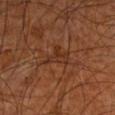workup — no biopsy performed (imaged during a skin exam); image — total-body-photography crop, ~15 mm field of view; patient — male, roughly 60 years of age; tile lighting — cross-polarized illumination; site — the left forearm; diameter — ≈3.5 mm.